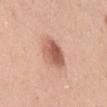notes: total-body-photography surveillance lesion; no biopsy
site: the left upper arm
image source: total-body-photography crop, ~15 mm field of view
patient: female, roughly 35 years of age